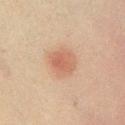- biopsy status — imaged on a skin check; not biopsied
- image source — 15 mm crop, total-body photography
- patient — male, aged approximately 45
- site — the right lower leg
- tile lighting — cross-polarized
- TBP lesion metrics — a lesion area of about 9.5 mm² and two-axis asymmetry of about 0.2
- lesion diameter — ~3.5 mm (longest diameter)